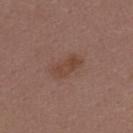Case summary:
– patient — female, aged around 45
– lighting — white-light illumination
– body site — the upper back
– acquisition — ~15 mm tile from a whole-body skin photo
– lesion size — about 4 mm